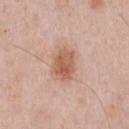notes — catalogued during a skin exam; not biopsied
patient — male, aged around 60
location — the chest
image — ~15 mm crop, total-body skin-cancer survey